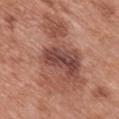A 15 mm crop from a total-body photograph taken for skin-cancer surveillance. The total-body-photography lesion software estimated a footprint of about 42 mm², an outline eccentricity of about 0.85 (0 = round, 1 = elongated), and a symmetry-axis asymmetry near 0.45. The analysis additionally found an average lesion color of about L≈50 a*≈23 b*≈27 (CIELAB), roughly 10 lightness units darker than nearby skin, and a normalized lesion–skin contrast near 7.5. The analysis additionally found a border-irregularity index near 6.5/10 and a within-lesion color-variation index near 7.5/10. The lesion is located on the mid back. The lesion's longest dimension is about 10.5 mm. The subject is a male roughly 70 years of age.